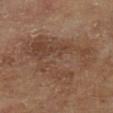  biopsy_status: not biopsied; imaged during a skin examination
  image:
    source: total-body photography crop
    field_of_view_mm: 15
  lesion_size:
    long_diameter_mm_approx: 9.0
  patient:
    sex: male
    age_approx: 65
  automated_metrics:
    cielab_L: 43
    cielab_a: 17
    cielab_b: 27
    vs_skin_contrast_norm: 5.5
    color_variation_0_10: 4.5
    peripheral_color_asymmetry: 1.5
  lighting: cross-polarized
  site: left lower leg